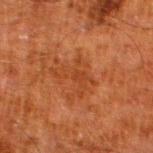Notes:
* follow-up: no biopsy performed (imaged during a skin exam)
* location: the left lower leg
* image-analysis metrics: a footprint of about 9 mm², an outline eccentricity of about 0.7 (0 = round, 1 = elongated), and a symmetry-axis asymmetry near 0.55
* patient: male, aged 78–82
* imaging modality: 15 mm crop, total-body photography